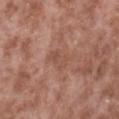No biopsy was performed on this lesion — it was imaged during a full skin examination and was not determined to be concerning. On the right lower leg. The subject is a male aged 53 to 57. Imaged with white-light lighting. Measured at roughly 4 mm in maximum diameter. A region of skin cropped from a whole-body photographic capture, roughly 15 mm wide. An algorithmic analysis of the crop reported a lesion color around L≈51 a*≈21 b*≈27 in CIELAB, a lesion–skin lightness drop of about 6, and a normalized lesion–skin contrast near 4.5.